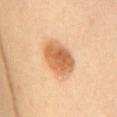A region of skin cropped from a whole-body photographic capture, roughly 15 mm wide. The lesion is on the mid back. A female patient, aged 38–42.